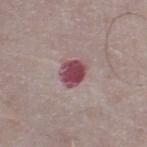notes = no biopsy performed (imaged during a skin exam).